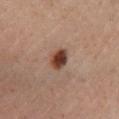Impression: Imaged during a routine full-body skin examination; the lesion was not biopsied and no histopathology is available. Acquisition and patient details: This is a cross-polarized tile. From the left lower leg. A male patient aged around 45. The lesion-visualizer software estimated a footprint of about 5.5 mm², an eccentricity of roughly 0.6, and a symmetry-axis asymmetry near 0.2. It also reported an average lesion color of about L≈38 a*≈20 b*≈26 (CIELAB), roughly 15 lightness units darker than nearby skin, and a normalized lesion–skin contrast near 12. Cropped from a whole-body photographic skin survey; the tile spans about 15 mm.